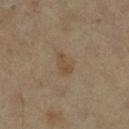{"biopsy_status": "not biopsied; imaged during a skin examination", "lesion_size": {"long_diameter_mm_approx": 3.0}, "site": "right lower leg", "image": {"source": "total-body photography crop", "field_of_view_mm": 15}, "automated_metrics": {"area_mm2_approx": 4.0, "eccentricity": 0.85, "shape_asymmetry": 0.35, "peripheral_color_asymmetry": 0.5}, "patient": {"age_approx": 60}}